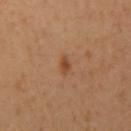{
  "biopsy_status": "not biopsied; imaged during a skin examination",
  "image": {
    "source": "total-body photography crop",
    "field_of_view_mm": 15
  },
  "site": "arm",
  "patient": {
    "sex": "female",
    "age_approx": 40
  },
  "lighting": "cross-polarized",
  "automated_metrics": {
    "area_mm2_approx": 2.0,
    "eccentricity": 0.7,
    "shape_asymmetry": 0.35,
    "cielab_L": 45,
    "cielab_a": 24,
    "cielab_b": 36,
    "vs_skin_contrast_norm": 8.0
  }
}